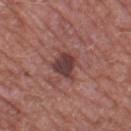Notes:
- workup — imaged on a skin check; not biopsied
- patient — male, aged around 60
- lesion diameter — ~3.5 mm (longest diameter)
- anatomic site — the leg
- acquisition — ~15 mm crop, total-body skin-cancer survey
- image-analysis metrics — border irregularity of about 2.5 on a 0–10 scale, a within-lesion color-variation index near 3/10, and peripheral color asymmetry of about 1; a classifier nevus-likeness of about 10/100 and lesion-presence confidence of about 100/100
- tile lighting — white-light illumination